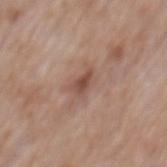The lesion was tiled from a total-body skin photograph and was not biopsied.
A close-up tile cropped from a whole-body skin photograph, about 15 mm across.
A male subject roughly 60 years of age.
About 2.5 mm across.
The total-body-photography lesion software estimated about 10 CIELAB-L* units darker than the surrounding skin and a lesion-to-skin contrast of about 7.5 (normalized; higher = more distinct). It also reported a border-irregularity index near 2.5/10, a color-variation rating of about 2.5/10, and peripheral color asymmetry of about 1. The analysis additionally found an automated nevus-likeness rating near 0 out of 100 and a detector confidence of about 100 out of 100 that the crop contains a lesion.
From the back.
This is a white-light tile.